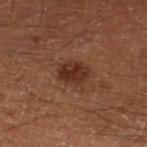Case summary:
* workup · imaged on a skin check; not biopsied
* size · about 3.5 mm
* patient · male, roughly 60 years of age
* image source · ~15 mm crop, total-body skin-cancer survey
* site · the left lower leg